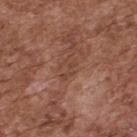<lesion>
  <lighting>white-light</lighting>
  <automated_metrics>
    <cielab_L>43</cielab_L>
    <cielab_a>21</cielab_a>
    <cielab_b>28</cielab_b>
    <vs_skin_contrast_norm>5.5</vs_skin_contrast_norm>
    <border_irregularity_0_10>6.5</border_irregularity_0_10>
    <peripheral_color_asymmetry>0.0</peripheral_color_asymmetry>
    <nevus_likeness_0_100>0</nevus_likeness_0_100>
    <lesion_detection_confidence_0_100>95</lesion_detection_confidence_0_100>
  </automated_metrics>
  <lesion_size>
    <long_diameter_mm_approx>3.0</long_diameter_mm_approx>
  </lesion_size>
  <patient>
    <sex>male</sex>
    <age_approx>75</age_approx>
  </patient>
  <image>
    <source>total-body photography crop</source>
    <field_of_view_mm>15</field_of_view_mm>
  </image>
  <site>upper back</site>
</lesion>